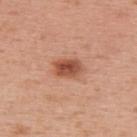Part of a total-body skin-imaging series; this lesion was reviewed on a skin check and was not flagged for biopsy.
Imaged with white-light lighting.
The lesion's longest dimension is about 3.5 mm.
A female patient, aged around 50.
The lesion is located on the upper back.
A 15 mm close-up extracted from a 3D total-body photography capture.